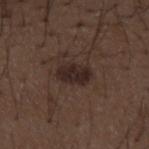Q: Was a biopsy performed?
A: imaged on a skin check; not biopsied
Q: Lesion location?
A: the mid back
Q: Patient demographics?
A: male, approximately 50 years of age
Q: How was the tile lit?
A: white-light illumination
Q: What kind of image is this?
A: ~15 mm crop, total-body skin-cancer survey
Q: How large is the lesion?
A: ≈3.5 mm